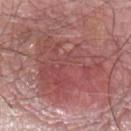Part of a total-body skin-imaging series; this lesion was reviewed on a skin check and was not flagged for biopsy. Longest diameter approximately 12.5 mm. The tile uses white-light illumination. The subject is a male about 55 years old. On the chest. The lesion-visualizer software estimated an area of roughly 50 mm², a shape eccentricity near 0.75, and two-axis asymmetry of about 0.45. It also reported a border-irregularity rating of about 8/10, a within-lesion color-variation index near 6/10, and peripheral color asymmetry of about 2. Cropped from a total-body skin-imaging series; the visible field is about 15 mm.